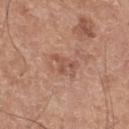biopsy status = no biopsy performed (imaged during a skin exam) | anatomic site = the left lower leg | image source = ~15 mm crop, total-body skin-cancer survey | automated metrics = a footprint of about 3.5 mm², a shape eccentricity near 0.8, and a symmetry-axis asymmetry near 0.6; a lesion–skin lightness drop of about 8 and a normalized lesion–skin contrast near 6; border irregularity of about 7 on a 0–10 scale, a color-variation rating of about 0.5/10, and peripheral color asymmetry of about 0 | subject = male, aged around 60.